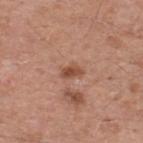A roughly 15 mm field-of-view crop from a total-body skin photograph.
On the upper back.
Captured under white-light illumination.
Longest diameter approximately 2.5 mm.
The subject is a male roughly 55 years of age.